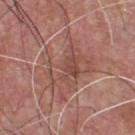follow-up: imaged on a skin check; not biopsied
anatomic site: the chest
image-analysis metrics: a footprint of about 25 mm²; about 7 CIELAB-L* units darker than the surrounding skin and a normalized lesion–skin contrast near 5
image source: 15 mm crop, total-body photography
lesion size: ~6.5 mm (longest diameter)
tile lighting: white-light illumination
patient: male, in their mid- to late 50s